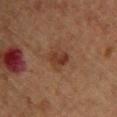The lesion was photographed on a routine skin check and not biopsied; there is no pathology result. The lesion's longest dimension is about 3 mm. The tile uses cross-polarized illumination. A 15 mm close-up extracted from a 3D total-body photography capture. The total-body-photography lesion software estimated border irregularity of about 2.5 on a 0–10 scale, a within-lesion color-variation index near 4/10, and peripheral color asymmetry of about 1.5. The lesion is located on the upper back. A female subject in their 70s.